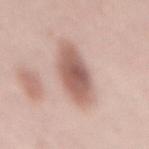{
  "biopsy_status": "not biopsied; imaged during a skin examination",
  "image": {
    "source": "total-body photography crop",
    "field_of_view_mm": 15
  },
  "patient": {
    "sex": "female",
    "age_approx": 60
  },
  "site": "mid back"
}